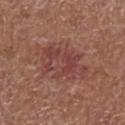Recorded during total-body skin imaging; not selected for excision or biopsy. Captured under white-light illumination. A male subject, aged 73 to 77. Approximately 6.5 mm at its widest. A 15 mm close-up extracted from a 3D total-body photography capture. On the right lower leg.